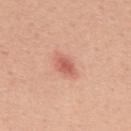follow-up: imaged on a skin check; not biopsied | image source: ~15 mm tile from a whole-body skin photo | anatomic site: the upper back | patient: male, aged approximately 55 | tile lighting: white-light illumination | size: ~3 mm (longest diameter).